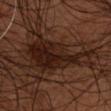Findings:
- follow-up — imaged on a skin check; not biopsied
- image-analysis metrics — border irregularity of about 8 on a 0–10 scale and a color-variation rating of about 7/10; a classifier nevus-likeness of about 50/100 and lesion-presence confidence of about 15/100
- location — the upper back
- imaging modality — ~15 mm crop, total-body skin-cancer survey
- lighting — cross-polarized
- lesion diameter — about 11 mm
- subject — male, aged approximately 45